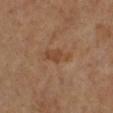A female subject aged approximately 65. From the right lower leg. Captured under cross-polarized illumination. A 15 mm crop from a total-body photograph taken for skin-cancer surveillance.